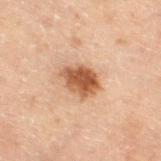{"biopsy_status": "not biopsied; imaged during a skin examination", "site": "right thigh", "automated_metrics": {"area_mm2_approx": 8.5, "eccentricity": 0.55, "shape_asymmetry": 0.15}, "lesion_size": {"long_diameter_mm_approx": 3.5}, "lighting": "cross-polarized", "patient": {"sex": "male", "age_approx": 70}, "image": {"source": "total-body photography crop", "field_of_view_mm": 15}}